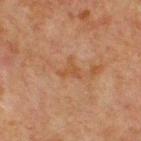| field | value |
|---|---|
| follow-up | catalogued during a skin exam; not biopsied |
| imaging modality | ~15 mm crop, total-body skin-cancer survey |
| subject | male, approximately 65 years of age |
| tile lighting | cross-polarized |
| automated lesion analysis | a footprint of about 3 mm² and a shape eccentricity near 0.45; a lesion–skin lightness drop of about 5 |
| size | ~2.5 mm (longest diameter) |
| body site | the front of the torso |